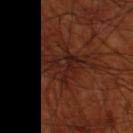The lesion was photographed on a routine skin check and not biopsied; there is no pathology result. A male patient, in their 70s. A roughly 15 mm field-of-view crop from a total-body skin photograph. Measured at roughly 4.5 mm in maximum diameter. From the leg.